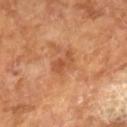No biopsy was performed on this lesion — it was imaged during a full skin examination and was not determined to be concerning.
Cropped from a whole-body photographic skin survey; the tile spans about 15 mm.
The patient is a male approximately 65 years of age.
Longest diameter approximately 3 mm.